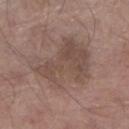{"image": {"source": "total-body photography crop", "field_of_view_mm": 15}, "patient": {"sex": "male", "age_approx": 80}, "lighting": "white-light", "site": "right lower leg"}